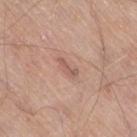Q: Is there a histopathology result?
A: imaged on a skin check; not biopsied
Q: How was the tile lit?
A: white-light illumination
Q: How was this image acquired?
A: ~15 mm tile from a whole-body skin photo
Q: Patient demographics?
A: male, aged around 80
Q: What is the anatomic site?
A: the right thigh